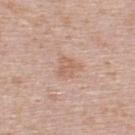Clinical impression: The lesion was tiled from a total-body skin photograph and was not biopsied. Background: The lesion is located on the upper back. A male patient aged approximately 40. The lesion's longest dimension is about 3 mm. Cropped from a total-body skin-imaging series; the visible field is about 15 mm. Imaged with white-light lighting.